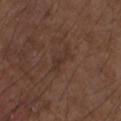Clinical impression: Captured during whole-body skin photography for melanoma surveillance; the lesion was not biopsied. Background: The tile uses white-light illumination. A male patient in their mid-70s. A 15 mm crop from a total-body photograph taken for skin-cancer surveillance. Located on the right forearm. An algorithmic analysis of the crop reported a border-irregularity rating of about 4/10, a color-variation rating of about 0.5/10, and radial color variation of about 0. And it measured a detector confidence of about 95 out of 100 that the crop contains a lesion.